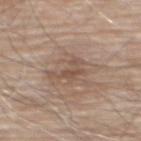Imaged during a routine full-body skin examination; the lesion was not biopsied and no histopathology is available. About 3.5 mm across. An algorithmic analysis of the crop reported a footprint of about 5 mm² and two-axis asymmetry of about 0.75. The analysis additionally found a lesion–skin lightness drop of about 8. The software also gave a color-variation rating of about 1/10 and peripheral color asymmetry of about 0. The subject is a male roughly 80 years of age. Imaged with white-light lighting. Located on the mid back. A lesion tile, about 15 mm wide, cut from a 3D total-body photograph.